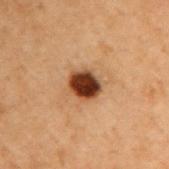A male patient roughly 70 years of age.
A region of skin cropped from a whole-body photographic capture, roughly 15 mm wide.
Located on the arm.